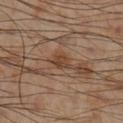Recorded during total-body skin imaging; not selected for excision or biopsy.
A male subject, in their mid-50s.
A region of skin cropped from a whole-body photographic capture, roughly 15 mm wide.
Captured under cross-polarized illumination.
On the right lower leg.